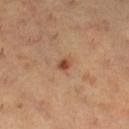Clinical impression: The lesion was photographed on a routine skin check and not biopsied; there is no pathology result. Image and clinical context: Longest diameter approximately 1.5 mm. Cropped from a total-body skin-imaging series; the visible field is about 15 mm. Automated tile analysis of the lesion measured an eccentricity of roughly 0.6 and two-axis asymmetry of about 0.2. The software also gave a lesion color around L≈47 a*≈24 b*≈35 in CIELAB, a lesion–skin lightness drop of about 12, and a normalized border contrast of about 9.5. The analysis additionally found a nevus-likeness score of about 90/100 and a lesion-detection confidence of about 100/100. A female subject in their 30s. From the right lower leg.